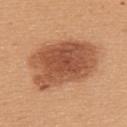Findings:
* notes: catalogued during a skin exam; not biopsied
* subject: female, aged 43 to 47
* diameter: ≈8 mm
* imaging modality: ~15 mm tile from a whole-body skin photo
* tile lighting: white-light illumination
* anatomic site: the upper back